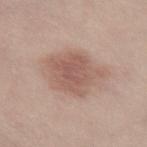No biopsy was performed on this lesion — it was imaged during a full skin examination and was not determined to be concerning.